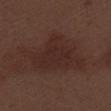Impression:
This lesion was catalogued during total-body skin photography and was not selected for biopsy.
Context:
The tile uses white-light illumination. The lesion's longest dimension is about 8.5 mm. Located on the right thigh. A region of skin cropped from a whole-body photographic capture, roughly 15 mm wide. A male subject, aged around 70.